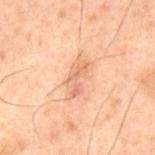{
  "biopsy_status": "not biopsied; imaged during a skin examination"
}